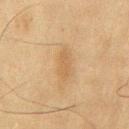subject: male, about 75 years old
lighting: cross-polarized
image source: total-body-photography crop, ~15 mm field of view
TBP lesion metrics: an automated nevus-likeness rating near 5 out of 100 and a lesion-detection confidence of about 100/100
diameter: ≈4 mm
body site: the chest